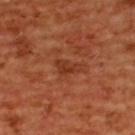biopsy_status: not biopsied; imaged during a skin examination
site: upper back
automated_metrics:
  eccentricity: 0.85
  shape_asymmetry: 0.35
  vs_skin_darker_L: 8.0
  vs_skin_contrast_norm: 6.5
  border_irregularity_0_10: 3.5
  color_variation_0_10: 1.5
patient:
  sex: female
  age_approx: 55
image:
  source: total-body photography crop
  field_of_view_mm: 15
lesion_size:
  long_diameter_mm_approx: 3.0